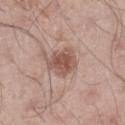Q: What did automated image analysis measure?
A: a mean CIELAB color near L≈53 a*≈20 b*≈25, a lesion–skin lightness drop of about 11, and a lesion-to-skin contrast of about 8 (normalized; higher = more distinct)
Q: How was this image acquired?
A: ~15 mm tile from a whole-body skin photo
Q: Where on the body is the lesion?
A: the right lower leg
Q: What lighting was used for the tile?
A: white-light
Q: Who is the patient?
A: male, in their mid- to late 30s
Q: How large is the lesion?
A: about 4 mm